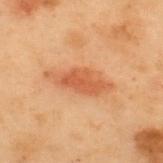Assessment: The lesion was photographed on a routine skin check and not biopsied; there is no pathology result. Clinical summary: A 15 mm crop from a total-body photograph taken for skin-cancer surveillance. Captured under cross-polarized illumination. Automated image analysis of the tile measured a within-lesion color-variation index near 2.5/10 and radial color variation of about 1. The software also gave a classifier nevus-likeness of about 100/100. Longest diameter approximately 6.5 mm. A male patient aged 53–57. The lesion is on the upper back.